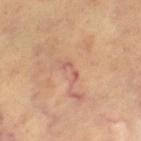Q: Was this lesion biopsied?
A: catalogued during a skin exam; not biopsied
Q: What did automated image analysis measure?
A: a mean CIELAB color near L≈59 a*≈24 b*≈28, about 7 CIELAB-L* units darker than the surrounding skin, and a lesion-to-skin contrast of about 5.5 (normalized; higher = more distinct); border irregularity of about 7 on a 0–10 scale, a within-lesion color-variation index near 0/10, and peripheral color asymmetry of about 0
Q: Who is the patient?
A: roughly 60 years of age
Q: What is the lesion's diameter?
A: ~3 mm (longest diameter)
Q: What kind of image is this?
A: ~15 mm crop, total-body skin-cancer survey
Q: Where on the body is the lesion?
A: the left thigh